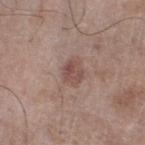Impression: Part of a total-body skin-imaging series; this lesion was reviewed on a skin check and was not flagged for biopsy. Image and clinical context: Located on the left lower leg. Captured under white-light illumination. Automated tile analysis of the lesion measured a shape eccentricity near 0.6 and a shape-asymmetry score of about 0.2 (0 = symmetric). Cropped from a total-body skin-imaging series; the visible field is about 15 mm. A male subject aged around 60. Measured at roughly 3 mm in maximum diameter.